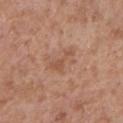This lesion was catalogued during total-body skin photography and was not selected for biopsy. A male subject in their mid- to late 70s. Measured at roughly 3.5 mm in maximum diameter. The lesion-visualizer software estimated a nevus-likeness score of about 0/100 and a lesion-detection confidence of about 100/100. Imaged with white-light lighting. Cropped from a total-body skin-imaging series; the visible field is about 15 mm. Located on the left lower leg.